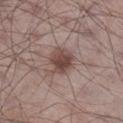* follow-up · catalogued during a skin exam; not biopsied
* patient · male, aged 53 to 57
* acquisition · 15 mm crop, total-body photography
* body site · the right lower leg